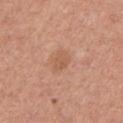Recorded during total-body skin imaging; not selected for excision or biopsy.
The patient is a male aged 43–47.
A 15 mm crop from a total-body photograph taken for skin-cancer surveillance.
Located on the upper back.